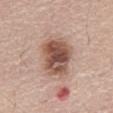A male subject, aged 58–62.
From the abdomen.
Cropped from a total-body skin-imaging series; the visible field is about 15 mm.
About 5.5 mm across.